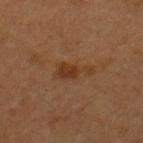Assessment:
Recorded during total-body skin imaging; not selected for excision or biopsy.
Acquisition and patient details:
On the upper back. The lesion's longest dimension is about 4 mm. The total-body-photography lesion software estimated a shape eccentricity near 0.9 and two-axis asymmetry of about 0.45. The software also gave roughly 6 lightness units darker than nearby skin and a normalized lesion–skin contrast near 7. The analysis additionally found a border-irregularity rating of about 5/10, internal color variation of about 1.5 on a 0–10 scale, and a peripheral color-asymmetry measure near 0.5. And it measured a nevus-likeness score of about 35/100. A close-up tile cropped from a whole-body skin photograph, about 15 mm across. The subject is a female aged approximately 40.